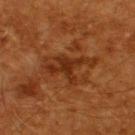| feature | finding |
|---|---|
| workup | catalogued during a skin exam; not biopsied |
| subject | male, approximately 60 years of age |
| location | the upper back |
| diameter | ~6.5 mm (longest diameter) |
| image source | ~15 mm tile from a whole-body skin photo |
| tile lighting | cross-polarized |